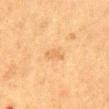This lesion was catalogued during total-body skin photography and was not selected for biopsy. A female subject, roughly 50 years of age. A roughly 15 mm field-of-view crop from a total-body skin photograph. From the abdomen. Approximately 2.5 mm at its widest. Imaged with cross-polarized lighting.